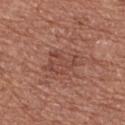Case summary:
* follow-up: catalogued during a skin exam; not biopsied
* subject: female, roughly 55 years of age
* body site: the upper back
* image: ~15 mm crop, total-body skin-cancer survey
* tile lighting: white-light illumination